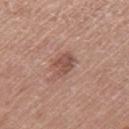The lesion was photographed on a routine skin check and not biopsied; there is no pathology result. A lesion tile, about 15 mm wide, cut from a 3D total-body photograph. From the right thigh. Automated image analysis of the tile measured a border-irregularity index near 3/10 and internal color variation of about 3 on a 0–10 scale. A female patient aged around 50. The recorded lesion diameter is about 3 mm. This is a white-light tile.